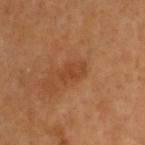{"biopsy_status": "not biopsied; imaged during a skin examination", "patient": {"sex": "male", "age_approx": 60}, "image": {"source": "total-body photography crop", "field_of_view_mm": 15}, "lesion_size": {"long_diameter_mm_approx": 3.0}, "lighting": "cross-polarized", "automated_metrics": {"cielab_L": 42, "cielab_a": 23, "cielab_b": 35, "vs_skin_darker_L": 7.0, "vs_skin_contrast_norm": 5.5, "nevus_likeness_0_100": 0, "lesion_detection_confidence_0_100": 100}, "site": "head or neck"}